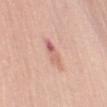This lesion was catalogued during total-body skin photography and was not selected for biopsy.
Cropped from a total-body skin-imaging series; the visible field is about 15 mm.
A female subject in their mid- to late 60s.
Approximately 3.5 mm at its widest.
Located on the left upper arm.
The lesion-visualizer software estimated a mean CIELAB color near L≈64 a*≈24 b*≈27, about 10 CIELAB-L* units darker than the surrounding skin, and a lesion-to-skin contrast of about 6 (normalized; higher = more distinct). It also reported a classifier nevus-likeness of about 0/100 and lesion-presence confidence of about 100/100.